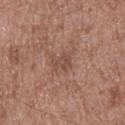Notes:
- workup — no biopsy performed (imaged during a skin exam)
- diameter — ~2.5 mm (longest diameter)
- tile lighting — white-light illumination
- body site — the mid back
- patient — male, about 50 years old
- automated metrics — an automated nevus-likeness rating near 0 out of 100 and lesion-presence confidence of about 100/100
- imaging modality — total-body-photography crop, ~15 mm field of view